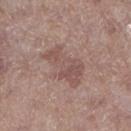{
  "biopsy_status": "not biopsied; imaged during a skin examination",
  "site": "left lower leg",
  "automated_metrics": {
    "cielab_L": 51,
    "cielab_a": 18,
    "cielab_b": 22,
    "vs_skin_darker_L": 8.0,
    "vs_skin_contrast_norm": 6.0,
    "border_irregularity_0_10": 4.5,
    "color_variation_0_10": 3.5,
    "peripheral_color_asymmetry": 1.0,
    "nevus_likeness_0_100": 0,
    "lesion_detection_confidence_0_100": 100
  },
  "lesion_size": {
    "long_diameter_mm_approx": 6.0
  },
  "patient": {
    "sex": "male",
    "age_approx": 80
  },
  "image": {
    "source": "total-body photography crop",
    "field_of_view_mm": 15
  },
  "lighting": "white-light"
}